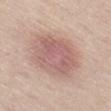| feature | finding |
|---|---|
| notes | total-body-photography surveillance lesion; no biopsy |
| automated lesion analysis | a footprint of about 24 mm² and a shape-asymmetry score of about 0.15 (0 = symmetric) |
| acquisition | ~15 mm crop, total-body skin-cancer survey |
| lighting | white-light |
| size | about 6.5 mm |
| site | the left thigh |
| subject | male, aged 58 to 62 |